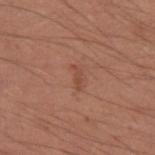biopsy status: total-body-photography surveillance lesion; no biopsy | diameter: ~2.5 mm (longest diameter) | automated metrics: a lesion area of about 2.5 mm², an eccentricity of roughly 0.9, and a shape-asymmetry score of about 0.5 (0 = symmetric); a color-variation rating of about 0/10 and peripheral color asymmetry of about 0; a nevus-likeness score of about 0/100 | site: the left upper arm | imaging modality: total-body-photography crop, ~15 mm field of view | subject: male, aged 33 to 37 | illumination: white-light illumination.